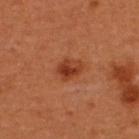Case summary:
- notes · catalogued during a skin exam; not biopsied
- subject · female, aged 48 to 52
- automated metrics · a lesion area of about 6 mm², an eccentricity of roughly 0.7, and two-axis asymmetry of about 0.2; an automated nevus-likeness rating near 95 out of 100 and a detector confidence of about 100 out of 100 that the crop contains a lesion
- tile lighting · cross-polarized
- imaging modality · ~15 mm crop, total-body skin-cancer survey